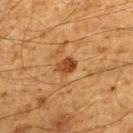{"biopsy_status": "not biopsied; imaged during a skin examination", "site": "upper back", "patient": {"sex": "male", "age_approx": 60}, "automated_metrics": {"vs_skin_darker_L": 11.0, "vs_skin_contrast_norm": 9.5}, "lighting": "cross-polarized", "image": {"source": "total-body photography crop", "field_of_view_mm": 15}}